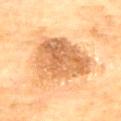| key | value |
|---|---|
| biopsy status | total-body-photography surveillance lesion; no biopsy |